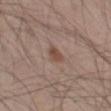No biopsy was performed on this lesion — it was imaged during a full skin examination and was not determined to be concerning.
The tile uses white-light illumination.
On the left thigh.
A male patient, in their mid- to late 40s.
Cropped from a whole-body photographic skin survey; the tile spans about 15 mm.